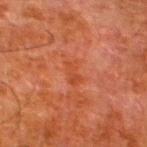Imaged during a routine full-body skin examination; the lesion was not biopsied and no histopathology is available.
A male subject, aged 78–82.
A 15 mm crop from a total-body photograph taken for skin-cancer surveillance.
The lesion is on the leg.